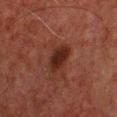Assessment:
The lesion was tiled from a total-body skin photograph and was not biopsied.
Background:
About 4 mm across. A male subject roughly 60 years of age. Automated image analysis of the tile measured an area of roughly 7.5 mm² and a symmetry-axis asymmetry near 0.2. And it measured an average lesion color of about L≈20 a*≈19 b*≈21 (CIELAB), a lesion–skin lightness drop of about 8, and a normalized border contrast of about 9.5. The lesion is on the chest. Imaged with cross-polarized lighting. A lesion tile, about 15 mm wide, cut from a 3D total-body photograph.